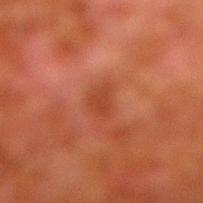Assessment: The lesion was tiled from a total-body skin photograph and was not biopsied. Context: The lesion is on the right lower leg. This is a cross-polarized tile. The lesion-visualizer software estimated a lesion–skin lightness drop of about 6 and a normalized border contrast of about 5.5. The software also gave an automated nevus-likeness rating near 0 out of 100 and lesion-presence confidence of about 100/100. A male subject, aged approximately 80. A 15 mm close-up extracted from a 3D total-body photography capture.